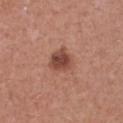Captured during whole-body skin photography for melanoma surveillance; the lesion was not biopsied.
The patient is a female aged 23–27.
A region of skin cropped from a whole-body photographic capture, roughly 15 mm wide.
The lesion is on the chest.
Automated image analysis of the tile measured a lesion area of about 6.5 mm² and an outline eccentricity of about 0.1 (0 = round, 1 = elongated). The software also gave an average lesion color of about L≈46 a*≈24 b*≈27 (CIELAB), about 13 CIELAB-L* units darker than the surrounding skin, and a normalized border contrast of about 9. The analysis additionally found an automated nevus-likeness rating near 85 out of 100 and a detector confidence of about 100 out of 100 that the crop contains a lesion.
Longest diameter approximately 3 mm.
The tile uses white-light illumination.